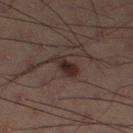Captured during whole-body skin photography for melanoma surveillance; the lesion was not biopsied.
Captured under cross-polarized illumination.
A 15 mm crop from a total-body photograph taken for skin-cancer surveillance.
A male patient approximately 60 years of age.
About 3.5 mm across.
Automated tile analysis of the lesion measured an eccentricity of roughly 0.8 and a shape-asymmetry score of about 0.3 (0 = symmetric). It also reported a lesion–skin lightness drop of about 8 and a normalized lesion–skin contrast near 9.5.